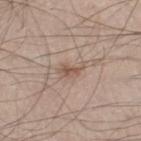| key | value |
|---|---|
| notes | imaged on a skin check; not biopsied |
| automated lesion analysis | a footprint of about 4 mm² and an outline eccentricity of about 0.85 (0 = round, 1 = elongated); a lesion color around L≈54 a*≈16 b*≈26 in CIELAB, a lesion–skin lightness drop of about 9, and a lesion-to-skin contrast of about 6.5 (normalized; higher = more distinct); a border-irregularity rating of about 3.5/10, a color-variation rating of about 2.5/10, and a peripheral color-asymmetry measure near 1 |
| site | the right lower leg |
| lesion size | ≈3 mm |
| image source | ~15 mm tile from a whole-body skin photo |
| tile lighting | white-light illumination |
| patient | male, in their mid- to late 20s |